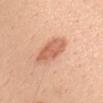biopsy status — total-body-photography surveillance lesion; no biopsy
subject — male, aged 38–42
acquisition — ~15 mm tile from a whole-body skin photo
site — the chest
lesion size — about 4.5 mm